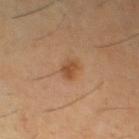biopsy status: catalogued during a skin exam; not biopsied.